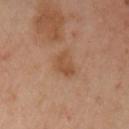follow-up=total-body-photography surveillance lesion; no biopsy